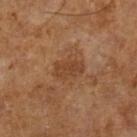Q: Is there a histopathology result?
A: catalogued during a skin exam; not biopsied
Q: Where on the body is the lesion?
A: the left lower leg
Q: Automated lesion metrics?
A: an area of roughly 7 mm² and an eccentricity of roughly 0.75; a mean CIELAB color near L≈41 a*≈20 b*≈32 and a normalized border contrast of about 6.5
Q: What is the lesion's diameter?
A: about 3.5 mm
Q: How was the tile lit?
A: cross-polarized
Q: Patient demographics?
A: male, about 65 years old
Q: How was this image acquired?
A: ~15 mm crop, total-body skin-cancer survey